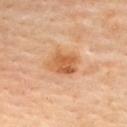Impression:
No biopsy was performed on this lesion — it was imaged during a full skin examination and was not determined to be concerning.
Image and clinical context:
Automated tile analysis of the lesion measured an automated nevus-likeness rating near 55 out of 100 and lesion-presence confidence of about 100/100. The lesion is located on the upper back. A female subject aged approximately 60. A 15 mm close-up tile from a total-body photography series done for melanoma screening. Imaged with cross-polarized lighting. The recorded lesion diameter is about 4 mm.